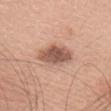Q: Was a biopsy performed?
A: total-body-photography surveillance lesion; no biopsy
Q: Where on the body is the lesion?
A: the head or neck
Q: What are the patient's age and sex?
A: female, aged 38 to 42
Q: What is the imaging modality?
A: ~15 mm tile from a whole-body skin photo